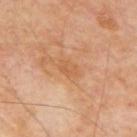follow-up = catalogued during a skin exam; not biopsied
image-analysis metrics = a mean CIELAB color near L≈58 a*≈22 b*≈38, roughly 6 lightness units darker than nearby skin, and a normalized border contrast of about 4.5; a border-irregularity index near 2/10, a color-variation rating of about 2/10, and peripheral color asymmetry of about 0.5
lighting = cross-polarized illumination
patient = male, approximately 70 years of age
size = ≈3 mm
imaging modality = ~15 mm tile from a whole-body skin photo
anatomic site = the mid back